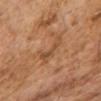| field | value |
|---|---|
| biopsy status | imaged on a skin check; not biopsied |
| lighting | cross-polarized illumination |
| anatomic site | the right upper arm |
| subject | female, in their 60s |
| TBP lesion metrics | a footprint of about 5 mm²; an average lesion color of about L≈42 a*≈19 b*≈31 (CIELAB) and a normalized lesion–skin contrast near 5.5 |
| imaging modality | ~15 mm tile from a whole-body skin photo |
| lesion diameter | ≈4 mm |